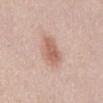Assessment: The lesion was tiled from a total-body skin photograph and was not biopsied. Context: The lesion's longest dimension is about 4 mm. Automated tile analysis of the lesion measured an area of roughly 8 mm². It also reported internal color variation of about 2.5 on a 0–10 scale and radial color variation of about 1. It also reported lesion-presence confidence of about 100/100. A roughly 15 mm field-of-view crop from a total-body skin photograph. A female patient, in their 30s. The lesion is located on the abdomen. Captured under white-light illumination.